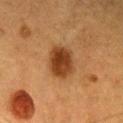Assessment:
Imaged during a routine full-body skin examination; the lesion was not biopsied and no histopathology is available.
Clinical summary:
The lesion is located on the head or neck. Captured under cross-polarized illumination. A female patient in their 40s. The recorded lesion diameter is about 4.5 mm. Cropped from a total-body skin-imaging series; the visible field is about 15 mm.